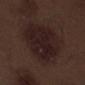Assessment: Imaged during a routine full-body skin examination; the lesion was not biopsied and no histopathology is available. Context: Cropped from a total-body skin-imaging series; the visible field is about 15 mm. Automated image analysis of the tile measured border irregularity of about 2.5 on a 0–10 scale, internal color variation of about 3.5 on a 0–10 scale, and a peripheral color-asymmetry measure near 1. Captured under white-light illumination. The subject is a male aged approximately 70. The recorded lesion diameter is about 8 mm. From the left thigh.